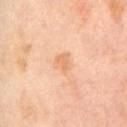Context: A male patient, roughly 65 years of age. A 15 mm close-up extracted from a 3D total-body photography capture. About 2.5 mm across. Imaged with cross-polarized lighting.